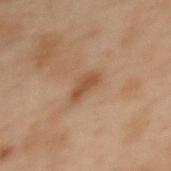follow-up: total-body-photography surveillance lesion; no biopsy | image source: ~15 mm crop, total-body skin-cancer survey | patient: female, aged 53 to 57 | anatomic site: the upper back.